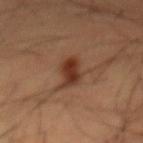biopsy status = catalogued during a skin exam; not biopsied | tile lighting = cross-polarized | site = the abdomen | lesion size = ~4 mm (longest diameter) | imaging modality = ~15 mm tile from a whole-body skin photo | patient = male, aged around 60.